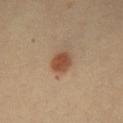Part of a total-body skin-imaging series; this lesion was reviewed on a skin check and was not flagged for biopsy.
Captured under cross-polarized illumination.
A female patient about 65 years old.
The lesion is located on the front of the torso.
About 3 mm across.
A 15 mm close-up extracted from a 3D total-body photography capture.
The total-body-photography lesion software estimated a mean CIELAB color near L≈47 a*≈20 b*≈31, a lesion–skin lightness drop of about 11, and a lesion-to-skin contrast of about 9 (normalized; higher = more distinct). The analysis additionally found a border-irregularity index near 2.5/10, a within-lesion color-variation index near 3/10, and peripheral color asymmetry of about 1.